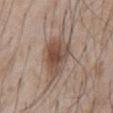Case summary:
• biopsy status — total-body-photography surveillance lesion; no biopsy